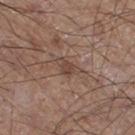Clinical impression: Part of a total-body skin-imaging series; this lesion was reviewed on a skin check and was not flagged for biopsy. Clinical summary: The tile uses white-light illumination. A 15 mm close-up tile from a total-body photography series done for melanoma screening. A male patient aged 58–62. From the right thigh.